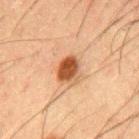follow-up: catalogued during a skin exam; not biopsied | image: total-body-photography crop, ~15 mm field of view | patient: male, in their 50s | body site: the mid back | tile lighting: cross-polarized illumination | lesion diameter: about 3.5 mm.